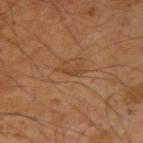  biopsy_status: not biopsied; imaged during a skin examination
  patient:
    sex: male
    age_approx: 50
  lighting: cross-polarized
  automated_metrics:
    area_mm2_approx: 2.0
    shape_asymmetry: 0.6
    cielab_L: 43
    cielab_a: 20
    cielab_b: 34
    vs_skin_contrast_norm: 5.5
    border_irregularity_0_10: 6.5
    color_variation_0_10: 0.0
    peripheral_color_asymmetry: 0.0
  lesion_size:
    long_diameter_mm_approx: 2.5
  image:
    source: total-body photography crop
    field_of_view_mm: 15
  site: left arm